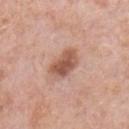Q: Was this lesion biopsied?
A: total-body-photography surveillance lesion; no biopsy
Q: Who is the patient?
A: male, in their 60s
Q: How was the tile lit?
A: white-light
Q: Lesion location?
A: the chest
Q: What kind of image is this?
A: 15 mm crop, total-body photography